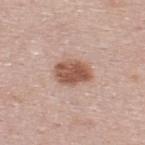No biopsy was performed on this lesion — it was imaged during a full skin examination and was not determined to be concerning. The subject is a male approximately 25 years of age. Automated tile analysis of the lesion measured a lesion area of about 9 mm² and a shape-asymmetry score of about 0.2 (0 = symmetric). And it measured a border-irregularity index near 1.5/10 and radial color variation of about 1.5. The analysis additionally found a classifier nevus-likeness of about 95/100 and a detector confidence of about 100 out of 100 that the crop contains a lesion. A 15 mm crop from a total-body photograph taken for skin-cancer surveillance. About 4 mm across. Imaged with white-light lighting. The lesion is on the upper back.